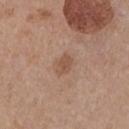{"biopsy_status": "not biopsied; imaged during a skin examination", "site": "chest", "lighting": "white-light", "image": {"source": "total-body photography crop", "field_of_view_mm": 15}, "patient": {"sex": "female", "age_approx": 35}, "lesion_size": {"long_diameter_mm_approx": 2.5}}